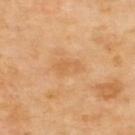Recorded during total-body skin imaging; not selected for excision or biopsy.
The tile uses cross-polarized illumination.
A close-up tile cropped from a whole-body skin photograph, about 15 mm across.
The lesion is located on the upper back.
An algorithmic analysis of the crop reported a lesion area of about 5.5 mm² and an outline eccentricity of about 0.8 (0 = round, 1 = elongated). The analysis additionally found a lesion color around L≈64 a*≈22 b*≈42 in CIELAB and a lesion-to-skin contrast of about 4.5 (normalized; higher = more distinct).